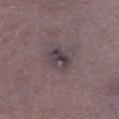Assessment: Captured during whole-body skin photography for melanoma surveillance; the lesion was not biopsied. Context: Approximately 3 mm at its widest. On the left lower leg. Imaged with white-light lighting. A roughly 15 mm field-of-view crop from a total-body skin photograph. A male subject, aged 48 to 52.